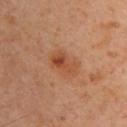Impression: This lesion was catalogued during total-body skin photography and was not selected for biopsy. Image and clinical context: A 15 mm crop from a total-body photograph taken for skin-cancer surveillance. Located on the upper back. The lesion-visualizer software estimated a lesion area of about 9.5 mm², an outline eccentricity of about 0.75 (0 = round, 1 = elongated), and a symmetry-axis asymmetry near 0.1. It also reported a mean CIELAB color near L≈50 a*≈25 b*≈35, a lesion–skin lightness drop of about 8, and a normalized border contrast of about 6. The recorded lesion diameter is about 4 mm. A male subject, aged 48–52. This is a cross-polarized tile.